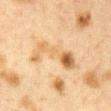Q: Is there a histopathology result?
A: imaged on a skin check; not biopsied
Q: What is the anatomic site?
A: the chest
Q: How was this image acquired?
A: ~15 mm crop, total-body skin-cancer survey
Q: Who is the patient?
A: female, roughly 40 years of age
Q: What is the lesion's diameter?
A: ~7 mm (longest diameter)